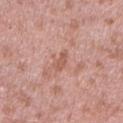workup: catalogued during a skin exam; not biopsied | anatomic site: the right upper arm | acquisition: ~15 mm crop, total-body skin-cancer survey | patient: male, in their 40s.